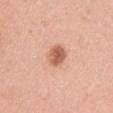Q: Is there a histopathology result?
A: no biopsy performed (imaged during a skin exam)
Q: How was the tile lit?
A: white-light
Q: How was this image acquired?
A: 15 mm crop, total-body photography
Q: How large is the lesion?
A: ≈3.5 mm
Q: What are the patient's age and sex?
A: female, aged around 35
Q: What did automated image analysis measure?
A: a border-irregularity rating of about 2/10, a color-variation rating of about 5.5/10, and peripheral color asymmetry of about 2
Q: Where on the body is the lesion?
A: the arm